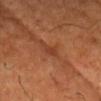| field | value |
|---|---|
| notes | no biopsy performed (imaged during a skin exam) |
| illumination | cross-polarized illumination |
| anatomic site | the head or neck |
| acquisition | ~15 mm tile from a whole-body skin photo |
| subject | male, aged around 40 |